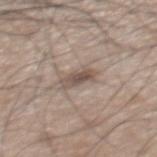anatomic site: the back | imaging modality: total-body-photography crop, ~15 mm field of view | illumination: white-light illumination | patient: male, aged around 75.